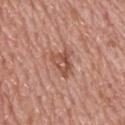biopsy status: catalogued during a skin exam; not biopsied
body site: the back
image source: total-body-photography crop, ~15 mm field of view
tile lighting: white-light illumination
patient: male, aged 73 to 77